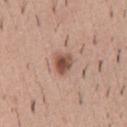Impression: The lesion was tiled from a total-body skin photograph and was not biopsied. Acquisition and patient details: A 15 mm crop from a total-body photograph taken for skin-cancer surveillance. From the chest. Longest diameter approximately 2.5 mm. The patient is a male in their 40s. This is a white-light tile.